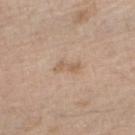Assessment:
Part of a total-body skin-imaging series; this lesion was reviewed on a skin check and was not flagged for biopsy.
Clinical summary:
A roughly 15 mm field-of-view crop from a total-body skin photograph. Approximately 3 mm at its widest. A male subject about 70 years old. The lesion is on the arm.